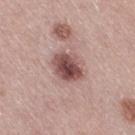image=~15 mm crop, total-body skin-cancer survey; subject=female, approximately 65 years of age; anatomic site=the right thigh.